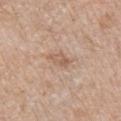Clinical impression:
Part of a total-body skin-imaging series; this lesion was reviewed on a skin check and was not flagged for biopsy.
Acquisition and patient details:
Measured at roughly 2.5 mm in maximum diameter. This is a white-light tile. A lesion tile, about 15 mm wide, cut from a 3D total-body photograph. Located on the chest. Automated image analysis of the tile measured a symmetry-axis asymmetry near 0.25. And it measured a nevus-likeness score of about 0/100. A male subject, approximately 70 years of age.